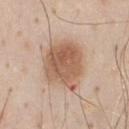follow-up: total-body-photography surveillance lesion; no biopsy | tile lighting: white-light illumination | anatomic site: the front of the torso | acquisition: 15 mm crop, total-body photography | diameter: ≈6 mm | patient: male, aged approximately 45.